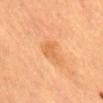| feature | finding |
|---|---|
| follow-up | imaged on a skin check; not biopsied |
| subject | female, about 55 years old |
| image source | total-body-photography crop, ~15 mm field of view |
| diameter | ≈3 mm |
| illumination | cross-polarized illumination |
| anatomic site | the mid back |
| image-analysis metrics | a footprint of about 6 mm²; a nevus-likeness score of about 0/100 and lesion-presence confidence of about 100/100 |